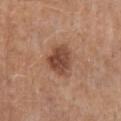The subject is a male in their 70s. About 3.5 mm across. The tile uses white-light illumination. From the mid back. A region of skin cropped from a whole-body photographic capture, roughly 15 mm wide.